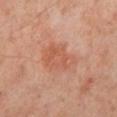site: left lower leg
lighting: cross-polarized
image:
  source: total-body photography crop
  field_of_view_mm: 15
lesion_size:
  long_diameter_mm_approx: 5.5
patient:
  sex: male
  age_approx: 50